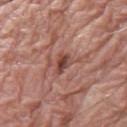Impression:
The lesion was photographed on a routine skin check and not biopsied; there is no pathology result.
Background:
A male patient approximately 80 years of age. A 15 mm close-up tile from a total-body photography series done for melanoma screening. The lesion is on the right lower leg.